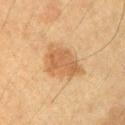Clinical impression: Part of a total-body skin-imaging series; this lesion was reviewed on a skin check and was not flagged for biopsy. Acquisition and patient details: The lesion is located on the right upper arm. A male subject aged 53–57. Imaged with cross-polarized lighting. An algorithmic analysis of the crop reported a mean CIELAB color near L≈56 a*≈19 b*≈37 and a normalized lesion–skin contrast near 6.5. And it measured a border-irregularity index near 3/10, a within-lesion color-variation index near 3/10, and peripheral color asymmetry of about 1. It also reported an automated nevus-likeness rating near 80 out of 100 and a detector confidence of about 100 out of 100 that the crop contains a lesion. The recorded lesion diameter is about 5.5 mm. A lesion tile, about 15 mm wide, cut from a 3D total-body photograph.